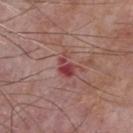Impression: Part of a total-body skin-imaging series; this lesion was reviewed on a skin check and was not flagged for biopsy. Clinical summary: A male patient, about 75 years old. Cropped from a total-body skin-imaging series; the visible field is about 15 mm. Located on the chest.